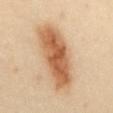Q: Is there a histopathology result?
A: no biopsy performed (imaged during a skin exam)
Q: Who is the patient?
A: female, aged approximately 60
Q: What lighting was used for the tile?
A: cross-polarized
Q: What kind of image is this?
A: ~15 mm crop, total-body skin-cancer survey
Q: What did automated image analysis measure?
A: a mean CIELAB color near L≈62 a*≈22 b*≈38, a lesion–skin lightness drop of about 15, and a lesion-to-skin contrast of about 9.5 (normalized; higher = more distinct); a border-irregularity rating of about 3/10, a color-variation rating of about 6.5/10, and a peripheral color-asymmetry measure near 2; a classifier nevus-likeness of about 100/100
Q: What is the lesion's diameter?
A: about 9 mm
Q: Lesion location?
A: the abdomen